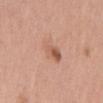follow-up=total-body-photography surveillance lesion; no biopsy | imaging modality=total-body-photography crop, ~15 mm field of view | diameter=about 4 mm | patient=female, aged 38 to 42 | site=the mid back | TBP lesion metrics=a lesion-detection confidence of about 100/100 | illumination=white-light.